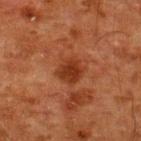Q: Was this lesion biopsied?
A: imaged on a skin check; not biopsied
Q: Where on the body is the lesion?
A: the upper back
Q: Patient demographics?
A: male, approximately 60 years of age
Q: What kind of image is this?
A: ~15 mm tile from a whole-body skin photo
Q: What did automated image analysis measure?
A: a classifier nevus-likeness of about 45/100 and a detector confidence of about 100 out of 100 that the crop contains a lesion
Q: Lesion size?
A: ~3 mm (longest diameter)